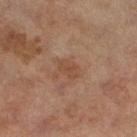Assessment:
Recorded during total-body skin imaging; not selected for excision or biopsy.
Clinical summary:
Imaged with cross-polarized lighting. A 15 mm crop from a total-body photograph taken for skin-cancer surveillance. Located on the right thigh. The recorded lesion diameter is about 2.5 mm. A female subject, roughly 60 years of age. Automated tile analysis of the lesion measured an area of roughly 3.5 mm², an outline eccentricity of about 0.75 (0 = round, 1 = elongated), and a symmetry-axis asymmetry near 0.25. And it measured a mean CIELAB color near L≈40 a*≈18 b*≈27, roughly 6 lightness units darker than nearby skin, and a normalized lesion–skin contrast near 5.5. It also reported a border-irregularity index near 3/10, internal color variation of about 1.5 on a 0–10 scale, and peripheral color asymmetry of about 0.5.